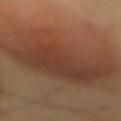Impression:
The lesion was tiled from a total-body skin photograph and was not biopsied.
Clinical summary:
Cropped from a whole-body photographic skin survey; the tile spans about 15 mm. The lesion's longest dimension is about 15.5 mm. On the mid back. A male subject, aged around 55. The tile uses cross-polarized illumination.